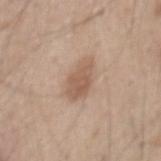The lesion was tiled from a total-body skin photograph and was not biopsied. The lesion is on the mid back. A male subject aged approximately 45. About 4.5 mm across. This image is a 15 mm lesion crop taken from a total-body photograph. Captured under white-light illumination.